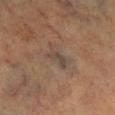biopsy_status: not biopsied; imaged during a skin examination
image:
  source: total-body photography crop
  field_of_view_mm: 15
site: left lower leg
lighting: cross-polarized
patient:
  sex: male
  age_approx: 70
lesion_size:
  long_diameter_mm_approx: 3.5